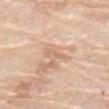Q: Was a biopsy performed?
A: catalogued during a skin exam; not biopsied
Q: What is the anatomic site?
A: the abdomen
Q: Who is the patient?
A: male, aged around 80
Q: How was this image acquired?
A: 15 mm crop, total-body photography
Q: What lighting was used for the tile?
A: white-light illumination
Q: Lesion size?
A: about 3 mm